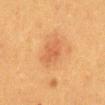Clinical impression:
This lesion was catalogued during total-body skin photography and was not selected for biopsy.
Background:
A female patient, roughly 40 years of age. This is a cross-polarized tile. A 15 mm crop from a total-body photograph taken for skin-cancer surveillance. The recorded lesion diameter is about 4 mm. On the chest.